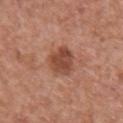Imaged during a routine full-body skin examination; the lesion was not biopsied and no histopathology is available. About 3.5 mm across. An algorithmic analysis of the crop reported an eccentricity of roughly 0.5. The software also gave a normalized lesion–skin contrast near 8. The analysis additionally found a classifier nevus-likeness of about 70/100 and a lesion-detection confidence of about 100/100. Cropped from a whole-body photographic skin survey; the tile spans about 15 mm. On the upper back. The patient is a male in their mid-40s.